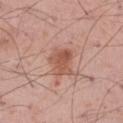illumination: white-light
image: ~15 mm tile from a whole-body skin photo
subject: male, in their mid-50s
anatomic site: the right thigh
diameter: ≈4 mm